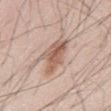follow-up: catalogued during a skin exam; not biopsied | TBP lesion metrics: a border-irregularity rating of about 3.5/10 and a color-variation rating of about 6/10 | subject: male, approximately 45 years of age | lighting: white-light | lesion diameter: ≈5 mm | image source: total-body-photography crop, ~15 mm field of view | anatomic site: the abdomen.